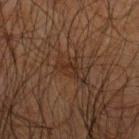This lesion was catalogued during total-body skin photography and was not selected for biopsy.
The subject is a male in their mid-60s.
An algorithmic analysis of the crop reported a footprint of about 3 mm² and a symmetry-axis asymmetry near 0.55.
The lesion is located on the left forearm.
This is a cross-polarized tile.
A lesion tile, about 15 mm wide, cut from a 3D total-body photograph.
About 3 mm across.